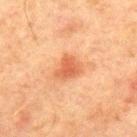Background:
The lesion is located on the chest. Automated image analysis of the tile measured a lesion–skin lightness drop of about 10 and a normalized border contrast of about 7. And it measured a classifier nevus-likeness of about 65/100 and a detector confidence of about 100 out of 100 that the crop contains a lesion. Captured under cross-polarized illumination. Approximately 3 mm at its widest. A male patient about 65 years old. A lesion tile, about 15 mm wide, cut from a 3D total-body photograph.